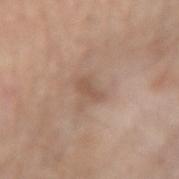Captured during whole-body skin photography for melanoma surveillance; the lesion was not biopsied. A male patient, aged 78–82. This image is a 15 mm lesion crop taken from a total-body photograph. The lesion-visualizer software estimated an area of roughly 3 mm², an eccentricity of roughly 0.85, and a shape-asymmetry score of about 0.4 (0 = symmetric). It also reported border irregularity of about 3.5 on a 0–10 scale, a color-variation rating of about 0.5/10, and radial color variation of about 0. The recorded lesion diameter is about 2.5 mm. From the right forearm. This is a white-light tile.